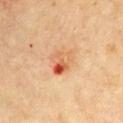Case summary:
• follow-up: imaged on a skin check; not biopsied
• image: 15 mm crop, total-body photography
• site: the chest
• subject: male, aged 68 to 72
• lesion size: ≈4 mm
• automated metrics: a mean CIELAB color near L≈59 a*≈26 b*≈38, a lesion–skin lightness drop of about 11, and a lesion-to-skin contrast of about 7 (normalized; higher = more distinct); a border-irregularity index near 3.5/10 and radial color variation of about 5.5; a classifier nevus-likeness of about 0/100 and a lesion-detection confidence of about 100/100